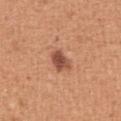workup: catalogued during a skin exam; not biopsied
patient: male, about 55 years old
body site: the abdomen
acquisition: ~15 mm tile from a whole-body skin photo